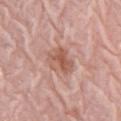{"biopsy_status": "not biopsied; imaged during a skin examination", "lesion_size": {"long_diameter_mm_approx": 4.0}, "site": "left arm", "lighting": "white-light", "automated_metrics": {"shape_asymmetry": 0.3, "border_irregularity_0_10": 3.0, "color_variation_0_10": 5.0, "peripheral_color_asymmetry": 1.5}, "patient": {"sex": "female", "age_approx": 65}, "image": {"source": "total-body photography crop", "field_of_view_mm": 15}}